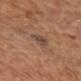This lesion was catalogued during total-body skin photography and was not selected for biopsy. Captured under white-light illumination. On the head or neck. The lesion-visualizer software estimated an eccentricity of roughly 0.75 and two-axis asymmetry of about 0.3. The analysis additionally found border irregularity of about 3.5 on a 0–10 scale, internal color variation of about 3.5 on a 0–10 scale, and a peripheral color-asymmetry measure near 1. The software also gave a nevus-likeness score of about 0/100 and a detector confidence of about 100 out of 100 that the crop contains a lesion. A female subject aged 73 to 77. This image is a 15 mm lesion crop taken from a total-body photograph. Longest diameter approximately 3.5 mm.